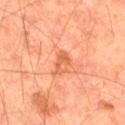Imaged during a routine full-body skin examination; the lesion was not biopsied and no histopathology is available. Captured under cross-polarized illumination. From the right thigh. A lesion tile, about 15 mm wide, cut from a 3D total-body photograph. The patient is a male approximately 65 years of age.